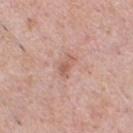Impression:
Part of a total-body skin-imaging series; this lesion was reviewed on a skin check and was not flagged for biopsy.